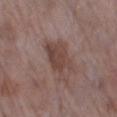– workup: catalogued during a skin exam; not biopsied
– image source: ~15 mm tile from a whole-body skin photo
– diameter: ~5.5 mm (longest diameter)
– lighting: white-light
– site: the leg
– patient: female, about 70 years old